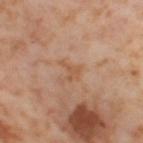This lesion was catalogued during total-body skin photography and was not selected for biopsy. A female subject, aged around 55. Longest diameter approximately 2.5 mm. A region of skin cropped from a whole-body photographic capture, roughly 15 mm wide. The lesion-visualizer software estimated a shape eccentricity near 0.75 and a symmetry-axis asymmetry near 0.6. The software also gave a color-variation rating of about 0/10 and radial color variation of about 0. From the left thigh.